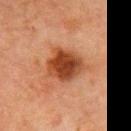Context:
A male subject in their 80s. Cropped from a whole-body photographic skin survey; the tile spans about 15 mm. Measured at roughly 5 mm in maximum diameter. Captured under cross-polarized illumination. The lesion is located on the abdomen.